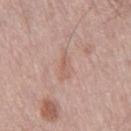follow-up: total-body-photography surveillance lesion; no biopsy | tile lighting: white-light | acquisition: ~15 mm tile from a whole-body skin photo | body site: the right thigh | automated metrics: a symmetry-axis asymmetry near 0.3; roughly 6 lightness units darker than nearby skin and a normalized lesion–skin contrast near 4.5; an automated nevus-likeness rating near 0 out of 100 and a detector confidence of about 100 out of 100 that the crop contains a lesion | patient: male, aged 73–77 | lesion diameter: ~4 mm (longest diameter).